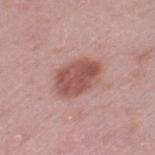The lesion was tiled from a total-body skin photograph and was not biopsied. Imaged with white-light lighting. A female subject, in their 40s. Automated tile analysis of the lesion measured a lesion color around L≈53 a*≈24 b*≈24 in CIELAB, about 12 CIELAB-L* units darker than the surrounding skin, and a lesion-to-skin contrast of about 8.5 (normalized; higher = more distinct). Measured at roughly 5 mm in maximum diameter. A 15 mm close-up extracted from a 3D total-body photography capture. Located on the left thigh.